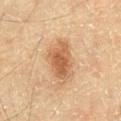No biopsy was performed on this lesion — it was imaged during a full skin examination and was not determined to be concerning. A male patient aged approximately 60. This image is a 15 mm lesion crop taken from a total-body photograph. The lesion's longest dimension is about 5.5 mm. Imaged with cross-polarized lighting.